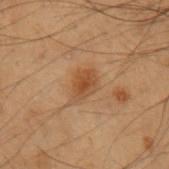– follow-up · imaged on a skin check; not biopsied
– illumination · cross-polarized
– lesion size · about 3 mm
– image-analysis metrics · a nevus-likeness score of about 65/100 and lesion-presence confidence of about 100/100
– imaging modality · ~15 mm crop, total-body skin-cancer survey
– subject · male, about 55 years old
– site · the left upper arm